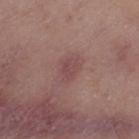{"biopsy_status": "not biopsied; imaged during a skin examination"}